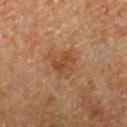notes: no biopsy performed (imaged during a skin exam) | subject: male, roughly 65 years of age | tile lighting: cross-polarized | lesion size: ~3.5 mm (longest diameter) | image: 15 mm crop, total-body photography | site: the arm.